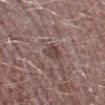The lesion was tiled from a total-body skin photograph and was not biopsied. The total-body-photography lesion software estimated an eccentricity of roughly 0.7 and two-axis asymmetry of about 0.25. The software also gave a lesion color around L≈43 a*≈17 b*≈19 in CIELAB, a lesion–skin lightness drop of about 8, and a normalized border contrast of about 7. The software also gave a within-lesion color-variation index near 3.5/10 and a peripheral color-asymmetry measure near 1. And it measured a nevus-likeness score of about 0/100 and lesion-presence confidence of about 70/100. A male patient in their mid- to late 40s. Located on the left lower leg. This image is a 15 mm lesion crop taken from a total-body photograph. Longest diameter approximately 2.5 mm. This is a white-light tile.